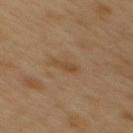Clinical impression:
Recorded during total-body skin imaging; not selected for excision or biopsy.
Background:
On the mid back. A male subject, aged around 50. Captured under cross-polarized illumination. A lesion tile, about 15 mm wide, cut from a 3D total-body photograph. The lesion-visualizer software estimated a lesion area of about 3 mm² and a symmetry-axis asymmetry near 0.4. And it measured a border-irregularity rating of about 4/10, internal color variation of about 0.5 on a 0–10 scale, and a peripheral color-asymmetry measure near 0.